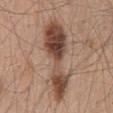follow-up: imaged on a skin check; not biopsied | TBP lesion metrics: a lesion area of about 24 mm² and an eccentricity of roughly 0.95; about 15 CIELAB-L* units darker than the surrounding skin and a normalized lesion–skin contrast near 10.5 | tile lighting: white-light | site: the mid back | diameter: ≈10 mm | patient: male, aged around 45 | image: 15 mm crop, total-body photography.